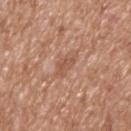Captured during whole-body skin photography for melanoma surveillance; the lesion was not biopsied.